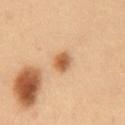Imaged during a routine full-body skin examination; the lesion was not biopsied and no histopathology is available. The patient is a male in their mid-50s. Automated image analysis of the tile measured a footprint of about 5 mm² and a shape-asymmetry score of about 0.2 (0 = symmetric). The analysis additionally found a mean CIELAB color near L≈48 a*≈18 b*≈32, about 11 CIELAB-L* units darker than the surrounding skin, and a normalized lesion–skin contrast near 8.5. It also reported a nevus-likeness score of about 95/100 and a lesion-detection confidence of about 100/100. On the abdomen. Cropped from a whole-body photographic skin survey; the tile spans about 15 mm. The recorded lesion diameter is about 2.5 mm.